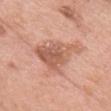From the head or neck.
The subject is a female approximately 60 years of age.
The recorded lesion diameter is about 6 mm.
Cropped from a total-body skin-imaging series; the visible field is about 15 mm.